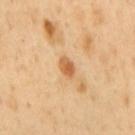Impression:
No biopsy was performed on this lesion — it was imaged during a full skin examination and was not determined to be concerning.
Background:
The total-body-photography lesion software estimated an area of roughly 3 mm², a shape eccentricity near 0.8, and a symmetry-axis asymmetry near 0.2. The analysis additionally found an average lesion color of about L≈60 a*≈24 b*≈41 (CIELAB) and about 13 CIELAB-L* units darker than the surrounding skin. The software also gave lesion-presence confidence of about 100/100. A male patient about 50 years old. The lesion is on the mid back. Imaged with cross-polarized lighting. A close-up tile cropped from a whole-body skin photograph, about 15 mm across. Longest diameter approximately 2.5 mm.